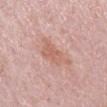Notes:
• notes · no biopsy performed (imaged during a skin exam)